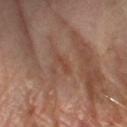Clinical impression:
No biopsy was performed on this lesion — it was imaged during a full skin examination and was not determined to be concerning.
Image and clinical context:
Cropped from a whole-body photographic skin survey; the tile spans about 15 mm. Located on the arm. A female patient aged 63–67.